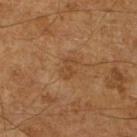Impression:
The lesion was photographed on a routine skin check and not biopsied; there is no pathology result.
Clinical summary:
A region of skin cropped from a whole-body photographic capture, roughly 15 mm wide. Captured under cross-polarized illumination. The patient is a male about 65 years old. The lesion's longest dimension is about 2.5 mm.